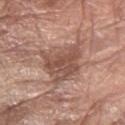No biopsy was performed on this lesion — it was imaged during a full skin examination and was not determined to be concerning.
About 6.5 mm across.
A female subject in their mid-70s.
A close-up tile cropped from a whole-body skin photograph, about 15 mm across.
Located on the right forearm.
This is a white-light tile.